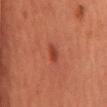biopsy status = no biopsy performed (imaged during a skin exam)
site = the mid back
patient = male, approximately 65 years of age
acquisition = ~15 mm crop, total-body skin-cancer survey
lighting = cross-polarized illumination
size = ~2.5 mm (longest diameter)
automated metrics = a border-irregularity index near 2.5/10, internal color variation of about 1 on a 0–10 scale, and a peripheral color-asymmetry measure near 0